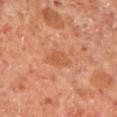Image and clinical context: The tile uses cross-polarized illumination. The total-body-photography lesion software estimated a border-irregularity rating of about 3/10 and a within-lesion color-variation index near 2.5/10. The lesion is located on the left lower leg. The subject is a male about 60 years old. A lesion tile, about 15 mm wide, cut from a 3D total-body photograph. Measured at roughly 3.5 mm in maximum diameter.